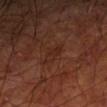Case summary:
• workup · no biopsy performed (imaged during a skin exam)
• site · the left upper arm
• illumination · cross-polarized illumination
• size · ~2.5 mm (longest diameter)
• patient · male, aged 63 to 67
• imaging modality · ~15 mm tile from a whole-body skin photo